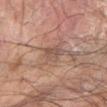Captured during whole-body skin photography for melanoma surveillance; the lesion was not biopsied.
The total-body-photography lesion software estimated border irregularity of about 3 on a 0–10 scale and peripheral color asymmetry of about 1. It also reported a nevus-likeness score of about 0/100 and lesion-presence confidence of about 50/100.
Cropped from a total-body skin-imaging series; the visible field is about 15 mm.
The lesion is on the right forearm.
The lesion's longest dimension is about 3.5 mm.
A male patient in their mid- to late 70s.